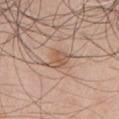{
  "biopsy_status": "not biopsied; imaged during a skin examination",
  "site": "chest",
  "automated_metrics": {
    "cielab_L": 56,
    "cielab_a": 19,
    "cielab_b": 30
  },
  "lighting": "white-light",
  "patient": {
    "sex": "male",
    "age_approx": 50
  },
  "image": {
    "source": "total-body photography crop",
    "field_of_view_mm": 15
  }
}